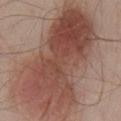Imaged during a routine full-body skin examination; the lesion was not biopsied and no histopathology is available. A close-up tile cropped from a whole-body skin photograph, about 15 mm across. Longest diameter approximately 14 mm. A male patient approximately 55 years of age. Imaged with white-light lighting. Automated tile analysis of the lesion measured a lesion color around L≈47 a*≈22 b*≈25 in CIELAB and roughly 13 lightness units darker than nearby skin. The software also gave an automated nevus-likeness rating near 100 out of 100 and a detector confidence of about 100 out of 100 that the crop contains a lesion. Located on the chest.